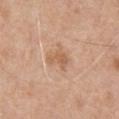<lesion>
  <biopsy_status>not biopsied; imaged during a skin examination</biopsy_status>
  <lesion_size>
    <long_diameter_mm_approx>3.0</long_diameter_mm_approx>
  </lesion_size>
  <automated_metrics>
    <area_mm2_approx>5.0</area_mm2_approx>
    <shape_asymmetry>0.4</shape_asymmetry>
    <border_irregularity_0_10>4.5</border_irregularity_0_10>
    <color_variation_0_10>2.0</color_variation_0_10>
    <peripheral_color_asymmetry>0.5</peripheral_color_asymmetry>
    <nevus_likeness_0_100>0</nevus_likeness_0_100>
    <lesion_detection_confidence_0_100>100</lesion_detection_confidence_0_100>
  </automated_metrics>
  <lighting>white-light</lighting>
  <image>
    <source>total-body photography crop</source>
    <field_of_view_mm>15</field_of_view_mm>
  </image>
  <patient>
    <sex>male</sex>
    <age_approx>65</age_approx>
  </patient>
  <site>front of the torso</site>
</lesion>